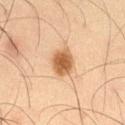Imaged with cross-polarized lighting. Approximately 3 mm at its widest. A male subject, roughly 65 years of age. The lesion is on the right thigh. A region of skin cropped from a whole-body photographic capture, roughly 15 mm wide.